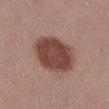Recorded during total-body skin imaging; not selected for excision or biopsy.
Approximately 5.5 mm at its widest.
A female patient, approximately 55 years of age.
On the left thigh.
Captured under white-light illumination.
A 15 mm close-up extracted from a 3D total-body photography capture.